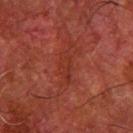biopsy status = catalogued during a skin exam; not biopsied | patient = male, approximately 80 years of age | location = the right forearm | automated lesion analysis = a mean CIELAB color near L≈26 a*≈25 b*≈25, a lesion–skin lightness drop of about 4, and a normalized border contrast of about 4.5; border irregularity of about 4 on a 0–10 scale and a within-lesion color-variation index near 0.5/10; a classifier nevus-likeness of about 0/100 and a detector confidence of about 55 out of 100 that the crop contains a lesion | imaging modality = 15 mm crop, total-body photography | tile lighting = cross-polarized illumination | diameter = ≈3.5 mm.